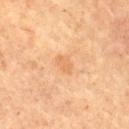biopsy status: total-body-photography surveillance lesion; no biopsy
illumination: cross-polarized illumination
size: ~2.5 mm (longest diameter)
acquisition: ~15 mm crop, total-body skin-cancer survey
location: the mid back
patient: male, in their mid-60s